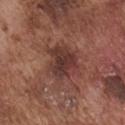Assessment: Captured during whole-body skin photography for melanoma surveillance; the lesion was not biopsied. Image and clinical context: On the front of the torso. A male patient approximately 75 years of age. A roughly 15 mm field-of-view crop from a total-body skin photograph. Longest diameter approximately 4.5 mm.